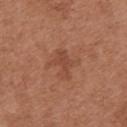Imaged during a routine full-body skin examination; the lesion was not biopsied and no histopathology is available. A female patient, in their mid-60s. A close-up tile cropped from a whole-body skin photograph, about 15 mm across. Imaged with white-light lighting. The lesion-visualizer software estimated a lesion area of about 6 mm², an outline eccentricity of about 0.85 (0 = round, 1 = elongated), and a shape-asymmetry score of about 0.4 (0 = symmetric). The analysis additionally found an average lesion color of about L≈46 a*≈24 b*≈31 (CIELAB), roughly 7 lightness units darker than nearby skin, and a normalized lesion–skin contrast near 5.5. The analysis additionally found lesion-presence confidence of about 100/100. The lesion is located on the arm. Measured at roughly 4 mm in maximum diameter.